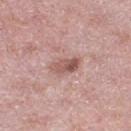- subject: female, about 40 years old
- site: the right thigh
- lighting: white-light
- image: ~15 mm tile from a whole-body skin photo